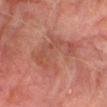Recorded during total-body skin imaging; not selected for excision or biopsy. The tile uses cross-polarized illumination. From the right lower leg. Cropped from a whole-body photographic skin survey; the tile spans about 15 mm. The lesion's longest dimension is about 9 mm. A male subject, approximately 75 years of age.